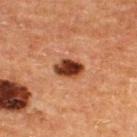| feature | finding |
|---|---|
| workup | total-body-photography surveillance lesion; no biopsy |
| illumination | cross-polarized |
| subject | male, aged approximately 65 |
| image | ~15 mm crop, total-body skin-cancer survey |
| automated lesion analysis | an area of roughly 6.5 mm², an eccentricity of roughly 0.75, and two-axis asymmetry of about 0.2; a color-variation rating of about 5.5/10; a classifier nevus-likeness of about 100/100 and a detector confidence of about 100 out of 100 that the crop contains a lesion |
| lesion size | ~3.5 mm (longest diameter) |
| location | the upper back |